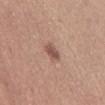Imaged during a routine full-body skin examination; the lesion was not biopsied and no histopathology is available.
The lesion is on the chest.
The tile uses white-light illumination.
A roughly 15 mm field-of-view crop from a total-body skin photograph.
The subject is a female aged 28 to 32.
Automated tile analysis of the lesion measured a footprint of about 4 mm², a shape eccentricity near 0.85, and two-axis asymmetry of about 0.25. The analysis additionally found an average lesion color of about L≈52 a*≈21 b*≈25 (CIELAB), roughly 11 lightness units darker than nearby skin, and a lesion-to-skin contrast of about 8 (normalized; higher = more distinct). The software also gave a border-irregularity rating of about 3/10, a within-lesion color-variation index near 2.5/10, and peripheral color asymmetry of about 0.5. The analysis additionally found a classifier nevus-likeness of about 80/100 and a lesion-detection confidence of about 100/100.